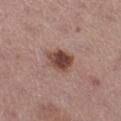Part of a total-body skin-imaging series; this lesion was reviewed on a skin check and was not flagged for biopsy.
A region of skin cropped from a whole-body photographic capture, roughly 15 mm wide.
Located on the left thigh.
A female patient aged approximately 55.